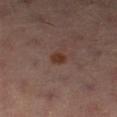lesion size = ≈2 mm | lighting = cross-polarized | image = ~15 mm crop, total-body skin-cancer survey | subject = female, aged 38 to 42 | automated lesion analysis = a footprint of about 3 mm², a shape eccentricity near 0.6, and a symmetry-axis asymmetry near 0.25; an automated nevus-likeness rating near 95 out of 100 and a lesion-detection confidence of about 100/100 | anatomic site = the left lower leg.